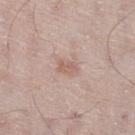Q: Was this lesion biopsied?
A: catalogued during a skin exam; not biopsied
Q: How was this image acquired?
A: ~15 mm crop, total-body skin-cancer survey
Q: What did automated image analysis measure?
A: an area of roughly 3.5 mm², a shape eccentricity near 0.8, and a symmetry-axis asymmetry near 0.3; an average lesion color of about L≈60 a*≈18 b*≈26 (CIELAB) and a lesion–skin lightness drop of about 8
Q: Illumination type?
A: white-light
Q: Lesion location?
A: the right thigh
Q: Who is the patient?
A: male, in their mid-60s